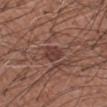The lesion was tiled from a total-body skin photograph and was not biopsied.
The recorded lesion diameter is about 2.5 mm.
Imaged with white-light lighting.
The subject is a male aged approximately 60.
From the left forearm.
A region of skin cropped from a whole-body photographic capture, roughly 15 mm wide.